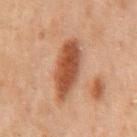biopsy status — total-body-photography surveillance lesion; no biopsy | tile lighting — cross-polarized | subject — male, roughly 70 years of age | imaging modality — ~15 mm crop, total-body skin-cancer survey | automated lesion analysis — border irregularity of about 1.5 on a 0–10 scale and a peripheral color-asymmetry measure near 1; a detector confidence of about 100 out of 100 that the crop contains a lesion | location — the mid back | lesion size — about 6 mm.